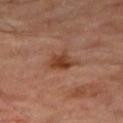Recorded during total-body skin imaging; not selected for excision or biopsy. The subject is a male roughly 70 years of age. Located on the right thigh. A region of skin cropped from a whole-body photographic capture, roughly 15 mm wide.